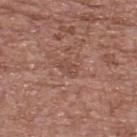workup: total-body-photography surveillance lesion; no biopsy | size: about 2.5 mm | image source: total-body-photography crop, ~15 mm field of view | automated lesion analysis: an area of roughly 3 mm², an eccentricity of roughly 0.85, and a shape-asymmetry score of about 0.3 (0 = symmetric); an average lesion color of about L≈47 a*≈21 b*≈26 (CIELAB), roughly 6 lightness units darker than nearby skin, and a normalized lesion–skin contrast near 5; a border-irregularity index near 2.5/10, internal color variation of about 1 on a 0–10 scale, and peripheral color asymmetry of about 0.5; a nevus-likeness score of about 0/100 and lesion-presence confidence of about 90/100 | body site: the back | patient: female, aged 63 to 67.